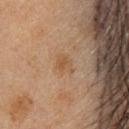Q: Patient demographics?
A: female, aged 43 to 47
Q: What kind of image is this?
A: ~15 mm crop, total-body skin-cancer survey
Q: How large is the lesion?
A: ≈2.5 mm
Q: What is the anatomic site?
A: the head or neck
Q: How was the tile lit?
A: cross-polarized illumination